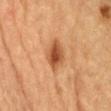On the front of the torso. The subject is a male aged around 85 or older. Cropped from a whole-body photographic skin survey; the tile spans about 15 mm. Approximately 3.5 mm at its widest.